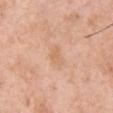<tbp_lesion>
<biopsy_status>not biopsied; imaged during a skin examination</biopsy_status>
<site>chest</site>
<lesion_size>
  <long_diameter_mm_approx>2.5</long_diameter_mm_approx>
</lesion_size>
<automated_metrics>
  <border_irregularity_0_10>3.0</border_irregularity_0_10>
  <color_variation_0_10>1.5</color_variation_0_10>
  <peripheral_color_asymmetry>0.5</peripheral_color_asymmetry>
</automated_metrics>
<lighting>white-light</lighting>
<image>
  <source>total-body photography crop</source>
  <field_of_view_mm>15</field_of_view_mm>
</image>
<patient>
  <sex>male</sex>
  <age_approx>55</age_approx>
</patient>
</tbp_lesion>